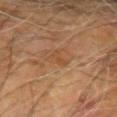{"image": {"source": "total-body photography crop", "field_of_view_mm": 15}, "lighting": "cross-polarized", "site": "left arm", "automated_metrics": {"border_irregularity_0_10": 5.0, "color_variation_0_10": 0.0, "peripheral_color_asymmetry": 0.0}, "lesion_size": {"long_diameter_mm_approx": 3.0}, "patient": {"sex": "male", "age_approx": 60}}